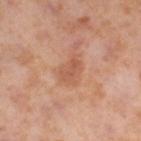This lesion was catalogued during total-body skin photography and was not selected for biopsy. A female patient, aged 53–57. A 15 mm close-up tile from a total-body photography series done for melanoma screening. The lesion is on the right lower leg. Captured under cross-polarized illumination. Longest diameter approximately 3.5 mm.